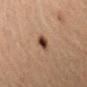Recorded during total-body skin imaging; not selected for excision or biopsy.
Automated image analysis of the tile measured a footprint of about 4.5 mm². It also reported a mean CIELAB color near L≈36 a*≈17 b*≈25, a lesion–skin lightness drop of about 13, and a lesion-to-skin contrast of about 11 (normalized; higher = more distinct). The analysis additionally found an automated nevus-likeness rating near 100 out of 100.
The tile uses cross-polarized illumination.
The recorded lesion diameter is about 2.5 mm.
Located on the right forearm.
A female subject roughly 40 years of age.
A roughly 15 mm field-of-view crop from a total-body skin photograph.